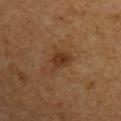Findings:
• workup: catalogued during a skin exam; not biopsied
• image source: ~15 mm crop, total-body skin-cancer survey
• automated lesion analysis: an area of roughly 5.5 mm² and an eccentricity of roughly 0.75; a nevus-likeness score of about 65/100 and lesion-presence confidence of about 100/100
• patient: male, aged approximately 60
• body site: the arm
• lesion size: ≈3.5 mm